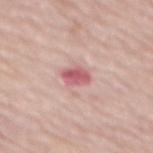No biopsy was performed on this lesion — it was imaged during a full skin examination and was not determined to be concerning. Automated image analysis of the tile measured roughly 13 lightness units darker than nearby skin and a lesion-to-skin contrast of about 8 (normalized; higher = more distinct). The analysis additionally found internal color variation of about 3.5 on a 0–10 scale. The software also gave a lesion-detection confidence of about 100/100. Cropped from a total-body skin-imaging series; the visible field is about 15 mm. The lesion's longest dimension is about 3 mm. A female subject aged around 65. On the back. Captured under white-light illumination.